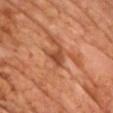workup: total-body-photography surveillance lesion; no biopsy | anatomic site: the chest | diameter: about 2.5 mm | subject: male, in their 70s | acquisition: ~15 mm tile from a whole-body skin photo | illumination: cross-polarized | image-analysis metrics: a mean CIELAB color near L≈47 a*≈27 b*≈35 and a normalized lesion–skin contrast near 7.5; a border-irregularity index near 4.5/10, internal color variation of about 2 on a 0–10 scale, and a peripheral color-asymmetry measure near 0.5; an automated nevus-likeness rating near 0 out of 100 and a lesion-detection confidence of about 100/100.